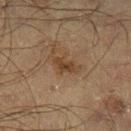biopsy_status: not biopsied; imaged during a skin examination
patient:
  sex: male
  age_approx: 50
image:
  source: total-body photography crop
  field_of_view_mm: 15
lighting: cross-polarized
site: left lower leg
automated_metrics:
  border_irregularity_0_10: 4.0
  peripheral_color_asymmetry: 1.0
lesion_size:
  long_diameter_mm_approx: 3.5